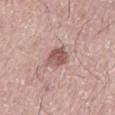This image is a 15 mm lesion crop taken from a total-body photograph. A male subject roughly 75 years of age. The tile uses white-light illumination. Located on the mid back.